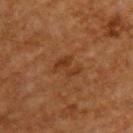Clinical impression: The lesion was photographed on a routine skin check and not biopsied; there is no pathology result. Acquisition and patient details: A male subject, roughly 60 years of age. The total-body-photography lesion software estimated a lesion area of about 5 mm², an eccentricity of roughly 0.75, and a shape-asymmetry score of about 0.55 (0 = symmetric). And it measured internal color variation of about 0.5 on a 0–10 scale and peripheral color asymmetry of about 0.5. It also reported a nevus-likeness score of about 0/100 and lesion-presence confidence of about 100/100. On the upper back. This is a cross-polarized tile. Cropped from a whole-body photographic skin survey; the tile spans about 15 mm. The recorded lesion diameter is about 3.5 mm.